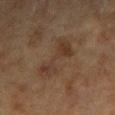From the arm. The lesion's longest dimension is about 6 mm. A roughly 15 mm field-of-view crop from a total-body skin photograph. A female subject, aged 78 to 82.